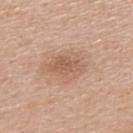This lesion was catalogued during total-body skin photography and was not selected for biopsy.
This is a white-light tile.
Measured at roughly 4.5 mm in maximum diameter.
A region of skin cropped from a whole-body photographic capture, roughly 15 mm wide.
Located on the upper back.
A male patient aged approximately 50.